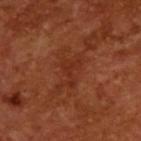Notes:
– follow-up · catalogued during a skin exam; not biopsied
– image · total-body-photography crop, ~15 mm field of view
– diameter · ~3.5 mm (longest diameter)
– lighting · cross-polarized
– patient · male, approximately 65 years of age
– TBP lesion metrics · an average lesion color of about L≈30 a*≈27 b*≈32 (CIELAB), roughly 5 lightness units darker than nearby skin, and a normalized border contrast of about 5; a nevus-likeness score of about 0/100 and a detector confidence of about 85 out of 100 that the crop contains a lesion